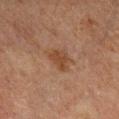Impression: Part of a total-body skin-imaging series; this lesion was reviewed on a skin check and was not flagged for biopsy. Clinical summary: A male subject aged around 65. The lesion-visualizer software estimated an area of roughly 6.5 mm², a shape eccentricity near 0.55, and two-axis asymmetry of about 0.35. And it measured a lesion color around L≈35 a*≈17 b*≈27 in CIELAB and about 6 CIELAB-L* units darker than the surrounding skin. On the left lower leg. Cropped from a whole-body photographic skin survey; the tile spans about 15 mm. This is a cross-polarized tile.